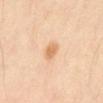Notes:
– workup: catalogued during a skin exam; not biopsied
– image source: 15 mm crop, total-body photography
– illumination: cross-polarized illumination
– patient: male, roughly 40 years of age
– site: the mid back
– lesion diameter: about 2.5 mm
– image-analysis metrics: an outline eccentricity of about 0.7 (0 = round, 1 = elongated) and a shape-asymmetry score of about 0.25 (0 = symmetric); a mean CIELAB color near L≈69 a*≈20 b*≈39, a lesion–skin lightness drop of about 10, and a lesion-to-skin contrast of about 7 (normalized; higher = more distinct); border irregularity of about 2.5 on a 0–10 scale, a within-lesion color-variation index near 1.5/10, and peripheral color asymmetry of about 0.5